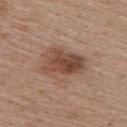Part of a total-body skin-imaging series; this lesion was reviewed on a skin check and was not flagged for biopsy. Captured under white-light illumination. A roughly 15 mm field-of-view crop from a total-body skin photograph. The lesion is located on the upper back. Automated image analysis of the tile measured a shape eccentricity near 0.8 and two-axis asymmetry of about 0.35. The subject is a female aged around 60. The lesion's longest dimension is about 5.5 mm.